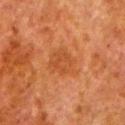Clinical summary: Measured at roughly 4 mm in maximum diameter. A 15 mm close-up extracted from a 3D total-body photography capture. The subject is a male approximately 80 years of age. Located on the left lower leg.